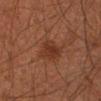Findings:
• biopsy status: no biopsy performed (imaged during a skin exam)
• body site: the arm
• subject: male, aged 43 to 47
• acquisition: ~15 mm tile from a whole-body skin photo